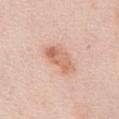workup: imaged on a skin check; not biopsied
TBP lesion metrics: a mean CIELAB color near L≈65 a*≈23 b*≈31, roughly 10 lightness units darker than nearby skin, and a normalized border contrast of about 7; a lesion-detection confidence of about 100/100
subject: female, roughly 65 years of age
tile lighting: white-light
size: ~4.5 mm (longest diameter)
body site: the abdomen
acquisition: ~15 mm crop, total-body skin-cancer survey